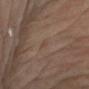Part of a total-body skin-imaging series; this lesion was reviewed on a skin check and was not flagged for biopsy. Cropped from a whole-body photographic skin survey; the tile spans about 15 mm. The lesion's longest dimension is about 1 mm. A female patient, in their mid-60s. The lesion is on the right upper arm.